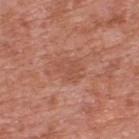No biopsy was performed on this lesion — it was imaged during a full skin examination and was not determined to be concerning.